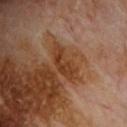Q: Was this lesion biopsied?
A: total-body-photography surveillance lesion; no biopsy
Q: What are the patient's age and sex?
A: male, aged 68 to 72
Q: Automated lesion metrics?
A: an outline eccentricity of about 0.9 (0 = round, 1 = elongated) and a shape-asymmetry score of about 0.4 (0 = symmetric); a mean CIELAB color near L≈38 a*≈22 b*≈33, a lesion–skin lightness drop of about 8, and a normalized border contrast of about 7.5; a nevus-likeness score of about 0/100 and lesion-presence confidence of about 100/100
Q: Where on the body is the lesion?
A: the chest
Q: What is the lesion's diameter?
A: about 4.5 mm
Q: Illumination type?
A: cross-polarized illumination
Q: What kind of image is this?
A: total-body-photography crop, ~15 mm field of view